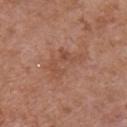Q: Was a biopsy performed?
A: catalogued during a skin exam; not biopsied
Q: What did automated image analysis measure?
A: a shape eccentricity near 0.85 and two-axis asymmetry of about 0.5; a mean CIELAB color near L≈49 a*≈23 b*≈30, about 7 CIELAB-L* units darker than the surrounding skin, and a normalized border contrast of about 5
Q: What lighting was used for the tile?
A: white-light illumination
Q: What is the imaging modality?
A: ~15 mm crop, total-body skin-cancer survey
Q: Patient demographics?
A: female, aged 38 to 42
Q: Lesion location?
A: the chest
Q: Lesion size?
A: ~4.5 mm (longest diameter)